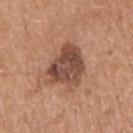{
  "biopsy_status": "not biopsied; imaged during a skin examination",
  "lesion_size": {
    "long_diameter_mm_approx": 5.5
  },
  "image": {
    "source": "total-body photography crop",
    "field_of_view_mm": 15
  },
  "automated_metrics": {
    "area_mm2_approx": 16.0,
    "shape_asymmetry": 0.3,
    "cielab_L": 47,
    "cielab_a": 21,
    "cielab_b": 28,
    "vs_skin_contrast_norm": 10.0
  },
  "patient": {
    "sex": "male",
    "age_approx": 75
  },
  "site": "right upper arm",
  "lighting": "white-light"
}